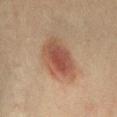{"biopsy_status": "not biopsied; imaged during a skin examination", "automated_metrics": {"area_mm2_approx": 16.0, "eccentricity": 0.7, "shape_asymmetry": 0.25, "cielab_L": 42, "cielab_a": 19, "cielab_b": 25, "vs_skin_darker_L": 10.0, "vs_skin_contrast_norm": 8.0}, "lesion_size": {"long_diameter_mm_approx": 5.5}, "image": {"source": "total-body photography crop", "field_of_view_mm": 15}, "lighting": "cross-polarized", "patient": {"sex": "female", "age_approx": 40}, "site": "lower back"}